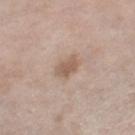Assessment:
No biopsy was performed on this lesion — it was imaged during a full skin examination and was not determined to be concerning.
Context:
A female patient, about 65 years old. The tile uses white-light illumination. The lesion is located on the right thigh. An algorithmic analysis of the crop reported a lesion area of about 4.5 mm² and an outline eccentricity of about 0.7 (0 = round, 1 = elongated). And it measured an automated nevus-likeness rating near 40 out of 100 and lesion-presence confidence of about 100/100. The lesion's longest dimension is about 2.5 mm. Cropped from a whole-body photographic skin survey; the tile spans about 15 mm.